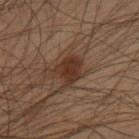Case summary:
• workup — catalogued during a skin exam; not biopsied
• image-analysis metrics — a footprint of about 7.5 mm², an outline eccentricity of about 0.55 (0 = round, 1 = elongated), and a symmetry-axis asymmetry near 0.25
• lesion diameter — ≈3 mm
• patient — male, aged around 55
• anatomic site — the chest
• imaging modality — ~15 mm tile from a whole-body skin photo
• lighting — cross-polarized illumination